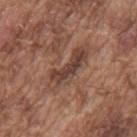Impression: Recorded during total-body skin imaging; not selected for excision or biopsy. Acquisition and patient details: The patient is a male in their mid-70s. This is a white-light tile. A 15 mm close-up extracted from a 3D total-body photography capture. The recorded lesion diameter is about 6 mm. Located on the mid back.